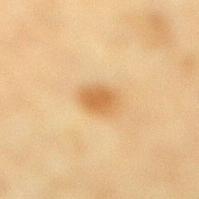The lesion was tiled from a total-body skin photograph and was not biopsied. From the right lower leg. A female patient, about 55 years old. A close-up tile cropped from a whole-body skin photograph, about 15 mm across. Imaged with cross-polarized lighting. The lesion's longest dimension is about 3 mm. Automated image analysis of the tile measured an area of roughly 6 mm², an eccentricity of roughly 0.6, and a shape-asymmetry score of about 0.2 (0 = symmetric). And it measured a mean CIELAB color near L≈54 a*≈17 b*≈38, roughly 9 lightness units darker than nearby skin, and a normalized border contrast of about 7.5. The analysis additionally found a border-irregularity rating of about 1.5/10, a within-lesion color-variation index near 2/10, and a peripheral color-asymmetry measure near 0.5. The software also gave a classifier nevus-likeness of about 90/100 and a detector confidence of about 100 out of 100 that the crop contains a lesion.